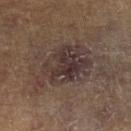<record>
  <biopsy_status>not biopsied; imaged during a skin examination</biopsy_status>
  <site>leg</site>
  <automated_metrics>
    <area_mm2_approx>24.0</area_mm2_approx>
    <eccentricity>0.8</eccentricity>
    <shape_asymmetry>0.35</shape_asymmetry>
    <border_irregularity_0_10>4.5</border_irregularity_0_10>
    <peripheral_color_asymmetry>2.0</peripheral_color_asymmetry>
    <nevus_likeness_0_100>0</nevus_likeness_0_100>
    <lesion_detection_confidence_0_100>100</lesion_detection_confidence_0_100>
  </automated_metrics>
  <lesion_size>
    <long_diameter_mm_approx>7.5</long_diameter_mm_approx>
  </lesion_size>
  <image>
    <source>total-body photography crop</source>
    <field_of_view_mm>15</field_of_view_mm>
  </image>
  <patient>
    <sex>male</sex>
    <age_approx>85+</age_approx>
  </patient>
</record>